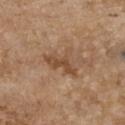Case summary:
• workup: imaged on a skin check; not biopsied
• subject: female, aged 63 to 67
• anatomic site: the chest
• image source: total-body-photography crop, ~15 mm field of view
• tile lighting: white-light